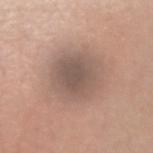Impression:
This lesion was catalogued during total-body skin photography and was not selected for biopsy.
Background:
The patient is a female in their mid-40s. Cropped from a total-body skin-imaging series; the visible field is about 15 mm. Longest diameter approximately 5.5 mm. The total-body-photography lesion software estimated a lesion area of about 21 mm², an eccentricity of roughly 0.5, and a shape-asymmetry score of about 0.15 (0 = symmetric). The analysis additionally found a border-irregularity index near 1.5/10, a color-variation rating of about 4/10, and a peripheral color-asymmetry measure near 1. The software also gave a detector confidence of about 95 out of 100 that the crop contains a lesion. From the right upper arm.